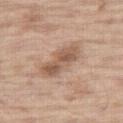notes: total-body-photography surveillance lesion; no biopsy | image: ~15 mm tile from a whole-body skin photo | automated metrics: a lesion area of about 11 mm² and an outline eccentricity of about 0.9 (0 = round, 1 = elongated); a nevus-likeness score of about 10/100 | tile lighting: white-light illumination | size: ≈5.5 mm | body site: the right thigh | subject: male, approximately 70 years of age.